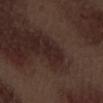Image and clinical context:
Automated tile analysis of the lesion measured a lesion color around L≈22 a*≈16 b*≈16 in CIELAB and a lesion-to-skin contrast of about 6.5 (normalized; higher = more distinct). It also reported a nevus-likeness score of about 0/100 and a lesion-detection confidence of about 95/100. A male patient, aged 68 to 72. A close-up tile cropped from a whole-body skin photograph, about 15 mm across. Imaged with white-light lighting. The lesion is on the left forearm. Approximately 3 mm at its widest.